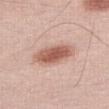  biopsy_status: not biopsied; imaged during a skin examination
  image:
    source: total-body photography crop
    field_of_view_mm: 15
  site: right thigh
  lighting: white-light
  lesion_size:
    long_diameter_mm_approx: 5.5
  patient:
    sex: male
    age_approx: 60